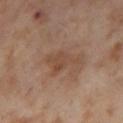{"biopsy_status": "not biopsied; imaged during a skin examination", "image": {"source": "total-body photography crop", "field_of_view_mm": 15}, "patient": {"sex": "female", "age_approx": 55}, "lesion_size": {"long_diameter_mm_approx": 4.5}, "lighting": "cross-polarized", "automated_metrics": {"shape_asymmetry": 0.45, "vs_skin_darker_L": 7.0, "vs_skin_contrast_norm": 6.0}, "site": "right thigh"}